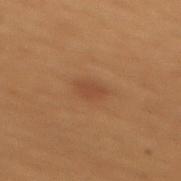The lesion was photographed on a routine skin check and not biopsied; there is no pathology result. This image is a 15 mm lesion crop taken from a total-body photograph. The total-body-photography lesion software estimated an area of roughly 4 mm², a shape eccentricity near 0.65, and a shape-asymmetry score of about 0.25 (0 = symmetric). A male subject aged 48 to 52. The tile uses cross-polarized illumination. Located on the mid back. The recorded lesion diameter is about 2.5 mm.